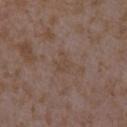Clinical impression: The lesion was photographed on a routine skin check and not biopsied; there is no pathology result. Background: A female patient, aged around 35. About 2.5 mm across. Located on the right forearm. An algorithmic analysis of the crop reported an eccentricity of roughly 0.65 and a symmetry-axis asymmetry near 0.4. The analysis additionally found about 4 CIELAB-L* units darker than the surrounding skin and a normalized lesion–skin contrast near 4.5. The software also gave border irregularity of about 5 on a 0–10 scale, a color-variation rating of about 1/10, and peripheral color asymmetry of about 0.5. A close-up tile cropped from a whole-body skin photograph, about 15 mm across.